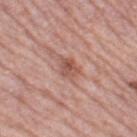No biopsy was performed on this lesion — it was imaged during a full skin examination and was not determined to be concerning. Imaged with white-light lighting. The lesion-visualizer software estimated about 10 CIELAB-L* units darker than the surrounding skin and a normalized border contrast of about 7. And it measured a border-irregularity index near 3/10, a color-variation rating of about 5/10, and peripheral color asymmetry of about 1.5. And it measured a classifier nevus-likeness of about 35/100 and a lesion-detection confidence of about 100/100. A 15 mm close-up extracted from a 3D total-body photography capture. On the leg. The patient is a female roughly 65 years of age. Measured at roughly 3 mm in maximum diameter.